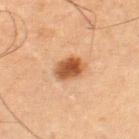notes: no biopsy performed (imaged during a skin exam) | image-analysis metrics: a shape eccentricity near 0.75 and a shape-asymmetry score of about 0.2 (0 = symmetric); border irregularity of about 2 on a 0–10 scale, a color-variation rating of about 4.5/10, and radial color variation of about 1.5; a lesion-detection confidence of about 100/100 | imaging modality: total-body-photography crop, ~15 mm field of view | location: the right thigh | subject: male, aged 63–67.